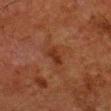follow-up — total-body-photography surveillance lesion; no biopsy
lesion size — about 4.5 mm
acquisition — 15 mm crop, total-body photography
lighting — cross-polarized
automated lesion analysis — a footprint of about 8 mm², a shape eccentricity near 0.75, and a shape-asymmetry score of about 0.5 (0 = symmetric); a lesion color around L≈28 a*≈20 b*≈26 in CIELAB; a border-irregularity index near 5.5/10 and a color-variation rating of about 3.5/10; a nevus-likeness score of about 0/100 and a lesion-detection confidence of about 100/100
anatomic site — the leg
patient — male, roughly 80 years of age